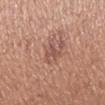<record>
<biopsy_status>not biopsied; imaged during a skin examination</biopsy_status>
<image>
  <source>total-body photography crop</source>
  <field_of_view_mm>15</field_of_view_mm>
</image>
<automated_metrics>
  <area_mm2_approx>3.0</area_mm2_approx>
  <eccentricity>0.8</eccentricity>
  <shape_asymmetry>0.55</shape_asymmetry>
  <cielab_L>52</cielab_L>
  <cielab_a>23</cielab_a>
  <cielab_b>26</cielab_b>
  <vs_skin_darker_L>8.0</vs_skin_darker_L>
  <border_irregularity_0_10>5.5</border_irregularity_0_10>
  <color_variation_0_10>0.0</color_variation_0_10>
  <peripheral_color_asymmetry>0.0</peripheral_color_asymmetry>
  <nevus_likeness_0_100>0</nevus_likeness_0_100>
  <lesion_detection_confidence_0_100>100</lesion_detection_confidence_0_100>
</automated_metrics>
<site>left forearm</site>
<lighting>white-light</lighting>
<patient>
  <sex>female</sex>
  <age_approx>55</age_approx>
</patient>
<lesion_size>
  <long_diameter_mm_approx>2.5</long_diameter_mm_approx>
</lesion_size>
</record>